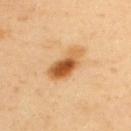<record>
<biopsy_status>not biopsied; imaged during a skin examination</biopsy_status>
<patient>
  <sex>male</sex>
  <age_approx>40</age_approx>
</patient>
<automated_metrics>
  <cielab_L>59</cielab_L>
  <cielab_a>24</cielab_a>
  <cielab_b>44</cielab_b>
  <vs_skin_contrast_norm>10.0</vs_skin_contrast_norm>
  <border_irregularity_0_10>2.5</border_irregularity_0_10>
  <nevus_likeness_0_100>100</nevus_likeness_0_100>
</automated_metrics>
<image>
  <source>total-body photography crop</source>
  <field_of_view_mm>15</field_of_view_mm>
</image>
<lighting>cross-polarized</lighting>
<lesion_size>
  <long_diameter_mm_approx>5.0</long_diameter_mm_approx>
</lesion_size>
<site>upper back</site>
</record>